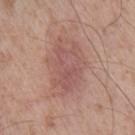workup = no biopsy performed (imaged during a skin exam) | lesion diameter = ≈6 mm | lighting = white-light | acquisition = 15 mm crop, total-body photography | automated metrics = a footprint of about 14 mm², a shape eccentricity near 0.8, and a symmetry-axis asymmetry near 0.35; a border-irregularity rating of about 5.5/10 and radial color variation of about 1; a classifier nevus-likeness of about 0/100 and lesion-presence confidence of about 100/100 | patient = male, about 55 years old | location = the front of the torso.